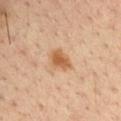notes: total-body-photography surveillance lesion; no biopsy
size: ≈3 mm
patient: male, aged approximately 35
acquisition: ~15 mm tile from a whole-body skin photo
lighting: cross-polarized illumination
location: the left upper arm